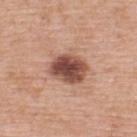biopsy_status: not biopsied; imaged during a skin examination
lesion_size:
  long_diameter_mm_approx: 4.0
image:
  source: total-body photography crop
  field_of_view_mm: 15
patient:
  sex: female
  age_approx: 40
lighting: white-light
site: upper back